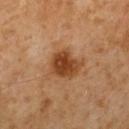The lesion was photographed on a routine skin check and not biopsied; there is no pathology result.
The tile uses cross-polarized illumination.
A 15 mm close-up extracted from a 3D total-body photography capture.
Located on the mid back.
Approximately 3.5 mm at its widest.
The patient is a male roughly 60 years of age.